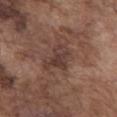| field | value |
|---|---|
| biopsy status | no biopsy performed (imaged during a skin exam) |
| anatomic site | the abdomen |
| lighting | white-light illumination |
| size | ~4 mm (longest diameter) |
| imaging modality | ~15 mm tile from a whole-body skin photo |
| patient | male, in their mid-70s |